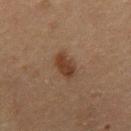<tbp_lesion>
  <biopsy_status>not biopsied; imaged during a skin examination</biopsy_status>
  <image>
    <source>total-body photography crop</source>
    <field_of_view_mm>15</field_of_view_mm>
  </image>
  <patient>
    <sex>male</sex>
    <age_approx>65</age_approx>
  </patient>
  <lighting>cross-polarized</lighting>
  <automated_metrics>
    <eccentricity>0.85</eccentricity>
    <shape_asymmetry>0.25</shape_asymmetry>
    <cielab_L>31</cielab_L>
    <cielab_a>16</cielab_a>
    <cielab_b>25</cielab_b>
    <vs_skin_darker_L>9.0</vs_skin_darker_L>
    <vs_skin_contrast_norm>8.5</vs_skin_contrast_norm>
    <border_irregularity_0_10>3.0</border_irregularity_0_10>
    <color_variation_0_10>2.5</color_variation_0_10>
    <peripheral_color_asymmetry>1.0</peripheral_color_asymmetry>
    <nevus_likeness_0_100>90</nevus_likeness_0_100>
    <lesion_detection_confidence_0_100>100</lesion_detection_confidence_0_100>
  </automated_metrics>
  <site>mid back</site>
</tbp_lesion>